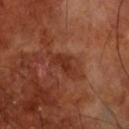<tbp_lesion>
  <biopsy_status>not biopsied; imaged during a skin examination</biopsy_status>
  <image>
    <source>total-body photography crop</source>
    <field_of_view_mm>15</field_of_view_mm>
  </image>
  <patient>
    <sex>male</sex>
    <age_approx>70</age_approx>
  </patient>
  <site>front of the torso</site>
  <lighting>cross-polarized</lighting>
  <lesion_size>
    <long_diameter_mm_approx>3.5</long_diameter_mm_approx>
  </lesion_size>
</tbp_lesion>